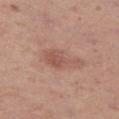<tbp_lesion>
  <biopsy_status>not biopsied; imaged during a skin examination</biopsy_status>
  <site>left thigh</site>
  <patient>
    <sex>female</sex>
    <age_approx>40</age_approx>
  </patient>
  <image>
    <source>total-body photography crop</source>
    <field_of_view_mm>15</field_of_view_mm>
  </image>
</tbp_lesion>